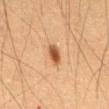Recorded during total-body skin imaging; not selected for excision or biopsy.
This image is a 15 mm lesion crop taken from a total-body photograph.
The lesion is located on the abdomen.
The lesion's longest dimension is about 3 mm.
The tile uses cross-polarized illumination.
A male patient, in their 50s.
The total-body-photography lesion software estimated a nevus-likeness score of about 100/100 and lesion-presence confidence of about 100/100.